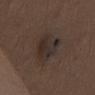| key | value |
|---|---|
| patient | male, in their 30s |
| image source | ~15 mm tile from a whole-body skin photo |
| automated lesion analysis | an eccentricity of roughly 0.85 and two-axis asymmetry of about 0.25; a mean CIELAB color near L≈28 a*≈10 b*≈17 and roughly 7 lightness units darker than nearby skin |
| diameter | ~5.5 mm (longest diameter) |
| anatomic site | the chest |